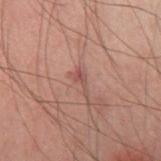{"biopsy_status": "not biopsied; imaged during a skin examination", "lighting": "cross-polarized", "lesion_size": {"long_diameter_mm_approx": 3.0}, "image": {"source": "total-body photography crop", "field_of_view_mm": 15}, "patient": {"sex": "male", "age_approx": 40}, "site": "right thigh"}